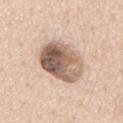Assessment:
Captured during whole-body skin photography for melanoma surveillance; the lesion was not biopsied.
Background:
Captured under white-light illumination. A roughly 15 mm field-of-view crop from a total-body skin photograph. The total-body-photography lesion software estimated a footprint of about 21 mm² and a shape-asymmetry score of about 0.1 (0 = symmetric). And it measured a mean CIELAB color near L≈59 a*≈16 b*≈27 and a normalized border contrast of about 11. And it measured a within-lesion color-variation index near 10/10 and a peripheral color-asymmetry measure near 4.5. The lesion is on the chest. About 6 mm across. A male subject aged around 50.